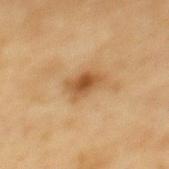follow-up=imaged on a skin check; not biopsied | patient=female, in their mid-50s | lighting=cross-polarized illumination | site=the mid back | acquisition=total-body-photography crop, ~15 mm field of view | lesion size=≈4 mm.